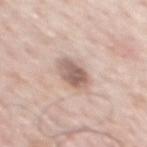Clinical impression: No biopsy was performed on this lesion — it was imaged during a full skin examination and was not determined to be concerning. Clinical summary: From the chest. A 15 mm close-up extracted from a 3D total-body photography capture. The recorded lesion diameter is about 3.5 mm. The tile uses white-light illumination. The patient is a male in their 60s.